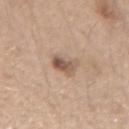Case summary:
* biopsy status · catalogued during a skin exam; not biopsied
* image source · 15 mm crop, total-body photography
* body site · the arm
* TBP lesion metrics · a border-irregularity rating of about 3.5/10, a color-variation rating of about 4/10, and radial color variation of about 1
* lighting · white-light illumination
* subject · female, roughly 45 years of age
* size · ~3 mm (longest diameter)